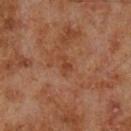The lesion was photographed on a routine skin check and not biopsied; there is no pathology result. Cropped from a total-body skin-imaging series; the visible field is about 15 mm. The lesion is located on the left lower leg. A male patient, aged approximately 70.